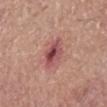Notes:
* follow-up: catalogued during a skin exam; not biopsied
* lighting: white-light
* body site: the left forearm
* diameter: ~4 mm (longest diameter)
* subject: female, aged 58 to 62
* image source: ~15 mm crop, total-body skin-cancer survey